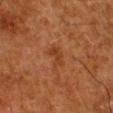<record>
<biopsy_status>not biopsied; imaged during a skin examination</biopsy_status>
<site>right lower leg</site>
<automated_metrics>
  <cielab_L>31</cielab_L>
  <cielab_a>21</cielab_a>
  <cielab_b>30</cielab_b>
  <vs_skin_contrast_norm>6.0</vs_skin_contrast_norm>
  <border_irregularity_0_10>4.5</border_irregularity_0_10>
  <color_variation_0_10>2.0</color_variation_0_10>
  <peripheral_color_asymmetry>0.5</peripheral_color_asymmetry>
  <nevus_likeness_0_100>0</nevus_likeness_0_100>
  <lesion_detection_confidence_0_100>100</lesion_detection_confidence_0_100>
</automated_metrics>
<lesion_size>
  <long_diameter_mm_approx>2.5</long_diameter_mm_approx>
</lesion_size>
<patient>
  <sex>male</sex>
  <age_approx>80</age_approx>
</patient>
<image>
  <source>total-body photography crop</source>
  <field_of_view_mm>15</field_of_view_mm>
</image>
<lighting>cross-polarized</lighting>
</record>